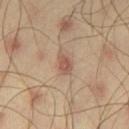  biopsy_status: not biopsied; imaged during a skin examination
  patient:
    sex: male
    age_approx: 45
  site: left thigh
  lesion_size:
    long_diameter_mm_approx: 3.0
  automated_metrics:
    border_irregularity_0_10: 3.0
    color_variation_0_10: 2.5
    peripheral_color_asymmetry: 1.0
    nevus_likeness_0_100: 25
  image:
    source: total-body photography crop
    field_of_view_mm: 15
  lighting: cross-polarized